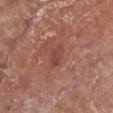notes: imaged on a skin check; not biopsied
image: ~15 mm crop, total-body skin-cancer survey
subject: male, roughly 70 years of age
TBP lesion metrics: a normalized border contrast of about 5.5; a border-irregularity rating of about 3/10, internal color variation of about 1.5 on a 0–10 scale, and a peripheral color-asymmetry measure near 0.5
illumination: white-light
anatomic site: the left lower leg
diameter: ≈2.5 mm